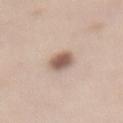workup = total-body-photography surveillance lesion; no biopsy | image source = ~15 mm crop, total-body skin-cancer survey | size = about 3.5 mm | illumination = white-light illumination | site = the back | subject = female, aged approximately 50.